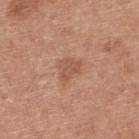Q: Is there a histopathology result?
A: imaged on a skin check; not biopsied